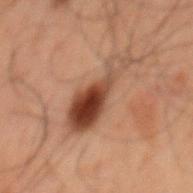* anatomic site: the mid back
* diameter: ~7.5 mm (longest diameter)
* illumination: cross-polarized
* subject: male, about 60 years old
* acquisition: ~15 mm crop, total-body skin-cancer survey